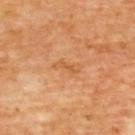workup: imaged on a skin check; not biopsied
patient: male, approximately 60 years of age
size: ≈3.5 mm
tile lighting: cross-polarized illumination
image source: total-body-photography crop, ~15 mm field of view
image-analysis metrics: a lesion color around L≈57 a*≈24 b*≈41 in CIELAB, a lesion–skin lightness drop of about 6, and a normalized lesion–skin contrast near 5; a border-irregularity index near 4.5/10, internal color variation of about 1 on a 0–10 scale, and radial color variation of about 0
body site: the upper back